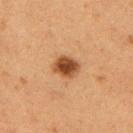The lesion was tiled from a total-body skin photograph and was not biopsied. From the upper back. The recorded lesion diameter is about 3 mm. The patient is a female about 40 years old. This is a cross-polarized tile. A 15 mm crop from a total-body photograph taken for skin-cancer surveillance.